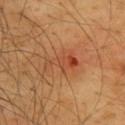Assessment: Part of a total-body skin-imaging series; this lesion was reviewed on a skin check and was not flagged for biopsy. Clinical summary: The tile uses cross-polarized illumination. Located on the upper back. Measured at roughly 4.5 mm in maximum diameter. Cropped from a whole-body photographic skin survey; the tile spans about 15 mm. A male patient roughly 65 years of age.